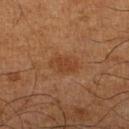Case summary:
• follow-up — catalogued during a skin exam; not biopsied
• automated lesion analysis — a footprint of about 7.5 mm² and a shape-asymmetry score of about 0.2 (0 = symmetric); internal color variation of about 1.5 on a 0–10 scale and radial color variation of about 0.5; a nevus-likeness score of about 5/100
• tile lighting — cross-polarized illumination
• image source — ~15 mm tile from a whole-body skin photo
• body site — the right lower leg
• subject — male, in their mid- to late 60s
• lesion size — about 4 mm